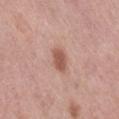Clinical impression:
The lesion was photographed on a routine skin check and not biopsied; there is no pathology result.
Context:
Automated tile analysis of the lesion measured a lesion area of about 5 mm², an outline eccentricity of about 0.8 (0 = round, 1 = elongated), and a shape-asymmetry score of about 0.2 (0 = symmetric). The software also gave a mean CIELAB color near L≈55 a*≈23 b*≈27, a lesion–skin lightness drop of about 12, and a normalized lesion–skin contrast near 8. And it measured a border-irregularity rating of about 2/10 and a color-variation rating of about 2/10. From the front of the torso. A 15 mm close-up extracted from a 3D total-body photography capture. Captured under white-light illumination. A female subject in their 60s.